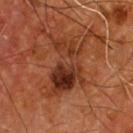No biopsy was performed on this lesion — it was imaged during a full skin examination and was not determined to be concerning. From the chest. A male patient, roughly 55 years of age. A 15 mm close-up tile from a total-body photography series done for melanoma screening. An algorithmic analysis of the crop reported a footprint of about 20 mm², a shape eccentricity near 0.85, and two-axis asymmetry of about 0.45. The software also gave a lesion color around L≈31 a*≈24 b*≈30 in CIELAB, a lesion–skin lightness drop of about 10, and a normalized lesion–skin contrast near 9. Captured under cross-polarized illumination.